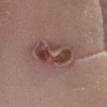<tbp_lesion>
  <biopsy_status>not biopsied; imaged during a skin examination</biopsy_status>
  <image>
    <source>total-body photography crop</source>
    <field_of_view_mm>15</field_of_view_mm>
  </image>
  <site>right lower leg</site>
  <patient>
    <sex>female</sex>
    <age_approx>55</age_approx>
  </patient>
</tbp_lesion>